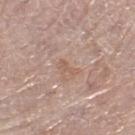Imaged with white-light lighting. A female patient in their mid-60s. The lesion is located on the right lower leg. Automated tile analysis of the lesion measured a lesion area of about 2 mm² and a symmetry-axis asymmetry near 0.45. The software also gave an average lesion color of about L≈58 a*≈18 b*≈28 (CIELAB), roughly 6 lightness units darker than nearby skin, and a normalized lesion–skin contrast near 5. The software also gave a border-irregularity rating of about 5/10 and a within-lesion color-variation index near 0/10. The recorded lesion diameter is about 2.5 mm. A 15 mm close-up extracted from a 3D total-body photography capture.